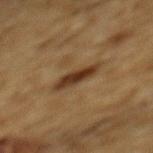On the mid back. Longest diameter approximately 5 mm. The subject is a male aged around 85 or older. A close-up tile cropped from a whole-body skin photograph, about 15 mm across.